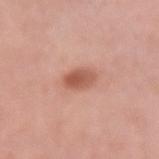Impression:
Recorded during total-body skin imaging; not selected for excision or biopsy.
Clinical summary:
Automated image analysis of the tile measured a footprint of about 6 mm², an outline eccentricity of about 0.7 (0 = round, 1 = elongated), and a shape-asymmetry score of about 0.1 (0 = symmetric). It also reported a mean CIELAB color near L≈56 a*≈26 b*≈30 and a normalized border contrast of about 8. It also reported a nevus-likeness score of about 90/100 and lesion-presence confidence of about 100/100. On the arm. A 15 mm crop from a total-body photograph taken for skin-cancer surveillance. Captured under white-light illumination. The subject is a female aged approximately 65. Approximately 3 mm at its widest.